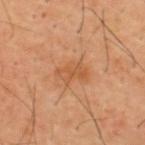Assessment: This lesion was catalogued during total-body skin photography and was not selected for biopsy. Image and clinical context: Automated tile analysis of the lesion measured a lesion area of about 6.5 mm², an outline eccentricity of about 0.8 (0 = round, 1 = elongated), and a symmetry-axis asymmetry near 0.35. A male subject, aged around 40. The tile uses cross-polarized illumination. A lesion tile, about 15 mm wide, cut from a 3D total-body photograph. On the upper back. The recorded lesion diameter is about 3.5 mm.